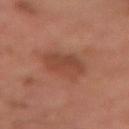Assessment:
The lesion was tiled from a total-body skin photograph and was not biopsied.